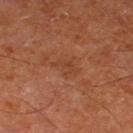Imaged during a routine full-body skin examination; the lesion was not biopsied and no histopathology is available.
Captured under cross-polarized illumination.
A 15 mm crop from a total-body photograph taken for skin-cancer surveillance.
The total-body-photography lesion software estimated a footprint of about 4.5 mm², a shape eccentricity near 0.8, and a symmetry-axis asymmetry near 0.4.
A male patient aged 63–67.
The recorded lesion diameter is about 3 mm.
On the left thigh.